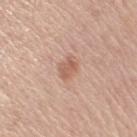Case summary:
* follow-up — catalogued during a skin exam; not biopsied
* location — the right upper arm
* patient — female, roughly 65 years of age
* tile lighting — white-light
* diameter — ~2.5 mm (longest diameter)
* image — ~15 mm tile from a whole-body skin photo